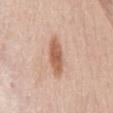Q: What did automated image analysis measure?
A: a border-irregularity rating of about 3/10, a color-variation rating of about 2.5/10, and radial color variation of about 0.5
Q: How large is the lesion?
A: ~5 mm (longest diameter)
Q: What lighting was used for the tile?
A: white-light
Q: What is the imaging modality?
A: 15 mm crop, total-body photography
Q: Patient demographics?
A: male, aged 58 to 62
Q: Lesion location?
A: the front of the torso